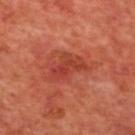This lesion was catalogued during total-body skin photography and was not selected for biopsy.
The lesion-visualizer software estimated about 8 CIELAB-L* units darker than the surrounding skin and a normalized lesion–skin contrast near 6. And it measured a border-irregularity rating of about 4.5/10 and radial color variation of about 2. It also reported a classifier nevus-likeness of about 0/100 and a lesion-detection confidence of about 100/100.
Captured under cross-polarized illumination.
The lesion is located on the mid back.
A close-up tile cropped from a whole-body skin photograph, about 15 mm across.
A male subject aged approximately 70.